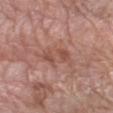Assessment:
This lesion was catalogued during total-body skin photography and was not selected for biopsy.
Clinical summary:
A female subject, approximately 70 years of age. The tile uses white-light illumination. A 15 mm close-up extracted from a 3D total-body photography capture. The lesion is on the arm. The recorded lesion diameter is about 5 mm.